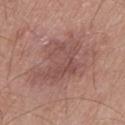Part of a total-body skin-imaging series; this lesion was reviewed on a skin check and was not flagged for biopsy.
A lesion tile, about 15 mm wide, cut from a 3D total-body photograph.
A male subject aged 78 to 82.
The total-body-photography lesion software estimated a lesion area of about 35 mm², an outline eccentricity of about 0.65 (0 = round, 1 = elongated), and two-axis asymmetry of about 0.3. The analysis additionally found an average lesion color of about L≈52 a*≈21 b*≈23 (CIELAB) and roughly 8 lightness units darker than nearby skin.
On the left thigh.